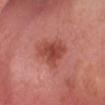Clinical impression:
No biopsy was performed on this lesion — it was imaged during a full skin examination and was not determined to be concerning.
Acquisition and patient details:
A male subject, aged 38–42. This image is a 15 mm lesion crop taken from a total-body photograph. The lesion is on the head or neck.